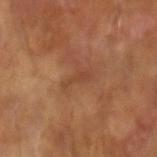<tbp_lesion>
<biopsy_status>not biopsied; imaged during a skin examination</biopsy_status>
<lesion_size>
  <long_diameter_mm_approx>3.0</long_diameter_mm_approx>
</lesion_size>
<patient>
  <sex>male</sex>
  <age_approx>65</age_approx>
</patient>
<lighting>cross-polarized</lighting>
<image>
  <source>total-body photography crop</source>
  <field_of_view_mm>15</field_of_view_mm>
</image>
</tbp_lesion>